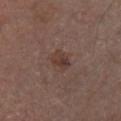• workup — total-body-photography surveillance lesion; no biopsy
• site — the front of the torso
• image — total-body-photography crop, ~15 mm field of view
• patient — male, aged 53–57